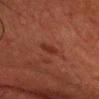Q: Is there a histopathology result?
A: total-body-photography surveillance lesion; no biopsy
Q: How was this image acquired?
A: 15 mm crop, total-body photography
Q: What did automated image analysis measure?
A: a mean CIELAB color near L≈25 a*≈22 b*≈24, about 5 CIELAB-L* units darker than the surrounding skin, and a normalized border contrast of about 6.5; a border-irregularity index near 2.5/10 and internal color variation of about 1 on a 0–10 scale; an automated nevus-likeness rating near 5 out of 100
Q: What are the patient's age and sex?
A: male, aged 58 to 62
Q: What is the lesion's diameter?
A: ≈2.5 mm
Q: What lighting was used for the tile?
A: cross-polarized
Q: What is the anatomic site?
A: the head or neck